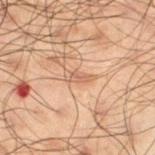biopsy status = imaged on a skin check; not biopsied | tile lighting = cross-polarized illumination | image source = ~15 mm crop, total-body skin-cancer survey | site = the left thigh | subject = male, aged around 50.